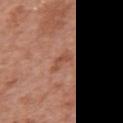{"biopsy_status": "not biopsied; imaged during a skin examination"}